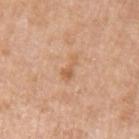notes = catalogued during a skin exam; not biopsied
body site = the left upper arm
lighting = white-light illumination
acquisition = 15 mm crop, total-body photography
subject = male, aged around 70
lesion size = about 3.5 mm